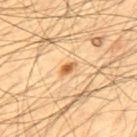The lesion was tiled from a total-body skin photograph and was not biopsied. A male subject, aged 53 to 57. Captured under cross-polarized illumination. A 15 mm close-up extracted from a 3D total-body photography capture. On the mid back. Longest diameter approximately 2 mm.